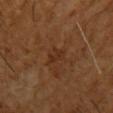Captured during whole-body skin photography for melanoma surveillance; the lesion was not biopsied.